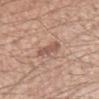  biopsy_status: not biopsied; imaged during a skin examination
  site: right upper arm
  patient:
    sex: male
    age_approx: 55
  lighting: white-light
  lesion_size:
    long_diameter_mm_approx: 2.5
  automated_metrics:
    border_irregularity_0_10: 3.0
    color_variation_0_10: 2.5
    peripheral_color_asymmetry: 1.0
  image:
    source: total-body photography crop
    field_of_view_mm: 15